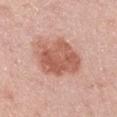| key | value |
|---|---|
| biopsy status | total-body-photography surveillance lesion; no biopsy |
| site | the left upper arm |
| subject | male, aged approximately 45 |
| image | total-body-photography crop, ~15 mm field of view |